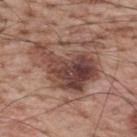– follow-up: catalogued during a skin exam; not biopsied
– site: the upper back
– lesion diameter: ~8 mm (longest diameter)
– subject: male, aged approximately 70
– image source: total-body-photography crop, ~15 mm field of view
– lighting: white-light